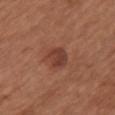Notes:
– workup · no biopsy performed (imaged during a skin exam)
– anatomic site · the chest
– lesion diameter · ≈3 mm
– patient · female, about 65 years old
– acquisition · 15 mm crop, total-body photography